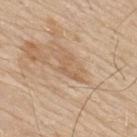Q: Was a biopsy performed?
A: catalogued during a skin exam; not biopsied
Q: Where on the body is the lesion?
A: the upper back
Q: What are the patient's age and sex?
A: male, approximately 80 years of age
Q: What is the lesion's diameter?
A: ~3.5 mm (longest diameter)
Q: What lighting was used for the tile?
A: white-light illumination
Q: What kind of image is this?
A: ~15 mm crop, total-body skin-cancer survey